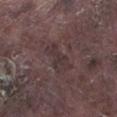biopsy status: total-body-photography surveillance lesion; no biopsy
image source: ~15 mm tile from a whole-body skin photo
patient: male, approximately 75 years of age
lesion diameter: ≈3.5 mm
tile lighting: white-light illumination
automated lesion analysis: a footprint of about 7 mm² and a shape eccentricity near 0.85; a mean CIELAB color near L≈33 a*≈14 b*≈14, about 5 CIELAB-L* units darker than the surrounding skin, and a normalized border contrast of about 5
location: the left lower leg